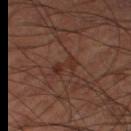acquisition = ~15 mm crop, total-body skin-cancer survey; illumination = cross-polarized; anatomic site = the right thigh; size = ~3.5 mm (longest diameter); patient = male, aged approximately 60.